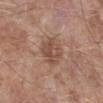Imaged during a routine full-body skin examination; the lesion was not biopsied and no histopathology is available. A male patient approximately 55 years of age. Captured under white-light illumination. A lesion tile, about 15 mm wide, cut from a 3D total-body photograph. The lesion-visualizer software estimated an average lesion color of about L≈49 a*≈20 b*≈26 (CIELAB) and roughly 9 lightness units darker than nearby skin. The software also gave an automated nevus-likeness rating near 0 out of 100 and a lesion-detection confidence of about 100/100. The lesion is on the left lower leg.